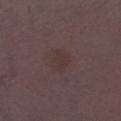Clinical impression:
This lesion was catalogued during total-body skin photography and was not selected for biopsy.
Context:
From the leg. A male patient about 50 years old. A lesion tile, about 15 mm wide, cut from a 3D total-body photograph.